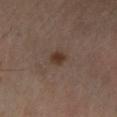Q: Was this lesion biopsied?
A: total-body-photography surveillance lesion; no biopsy